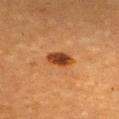Q: Is there a histopathology result?
A: no biopsy performed (imaged during a skin exam)
Q: What is the anatomic site?
A: the front of the torso
Q: How large is the lesion?
A: about 3.5 mm
Q: Who is the patient?
A: female, aged 53 to 57
Q: Automated lesion metrics?
A: an area of roughly 5.5 mm² and two-axis asymmetry of about 0.15
Q: What lighting was used for the tile?
A: cross-polarized illumination
Q: What is the imaging modality?
A: ~15 mm tile from a whole-body skin photo